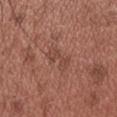workup: no biopsy performed (imaged during a skin exam); body site: the head or neck; patient: male, about 25 years old; size: ~4 mm (longest diameter); image source: 15 mm crop, total-body photography; illumination: white-light illumination.